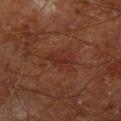biopsy_status: not biopsied; imaged during a skin examination
lighting: cross-polarized
site: leg
lesion_size:
  long_diameter_mm_approx: 3.5
automated_metrics:
  area_mm2_approx: 4.0
  eccentricity: 0.9
  shape_asymmetry: 0.3
  cielab_L: 28
  cielab_a: 24
  cielab_b: 26
  vs_skin_darker_L: 5.0
  vs_skin_contrast_norm: 5.5
  nevus_likeness_0_100: 0
  lesion_detection_confidence_0_100: 100
patient:
  sex: male
  age_approx: 65
image:
  source: total-body photography crop
  field_of_view_mm: 15